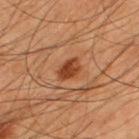Imaged during a routine full-body skin examination; the lesion was not biopsied and no histopathology is available.
Captured under cross-polarized illumination.
The lesion is on the upper back.
A male subject approximately 45 years of age.
A close-up tile cropped from a whole-body skin photograph, about 15 mm across.
Automated image analysis of the tile measured a border-irregularity index near 2/10 and radial color variation of about 1.5. It also reported a classifier nevus-likeness of about 95/100.
About 3 mm across.